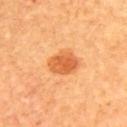Imaged during a routine full-body skin examination; the lesion was not biopsied and no histopathology is available. Located on the back. The subject is a male aged around 65. The tile uses cross-polarized illumination. Measured at roughly 3.5 mm in maximum diameter. The total-body-photography lesion software estimated an average lesion color of about L≈62 a*≈33 b*≈48 (CIELAB), a lesion–skin lightness drop of about 13, and a normalized border contrast of about 8. The analysis additionally found a border-irregularity rating of about 1.5/10, internal color variation of about 3 on a 0–10 scale, and peripheral color asymmetry of about 1. A roughly 15 mm field-of-view crop from a total-body skin photograph.